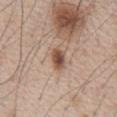Findings:
• image source: 15 mm crop, total-body photography
• anatomic site: the chest
• subject: male, in their mid- to late 60s
• lighting: white-light illumination